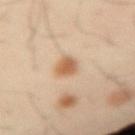Q: Was a biopsy performed?
A: imaged on a skin check; not biopsied
Q: What kind of image is this?
A: ~15 mm tile from a whole-body skin photo
Q: Automated lesion metrics?
A: an area of roughly 5 mm², a shape eccentricity near 0.7, and a symmetry-axis asymmetry near 0.25; a lesion color around L≈59 a*≈19 b*≈34 in CIELAB and a normalized lesion–skin contrast near 8.5; a nevus-likeness score of about 100/100 and a detector confidence of about 100 out of 100 that the crop contains a lesion
Q: What are the patient's age and sex?
A: male, aged around 40
Q: What lighting was used for the tile?
A: cross-polarized
Q: What is the lesion's diameter?
A: about 3 mm
Q: Lesion location?
A: the right upper arm